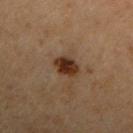The lesion was tiled from a total-body skin photograph and was not biopsied. Imaged with cross-polarized lighting. The lesion-visualizer software estimated a shape eccentricity near 0.45 and a shape-asymmetry score of about 0.2 (0 = symmetric). The analysis additionally found a lesion-detection confidence of about 100/100. From the arm. A 15 mm crop from a total-body photograph taken for skin-cancer surveillance. The lesion's longest dimension is about 2.5 mm. A male subject, aged 68–72.